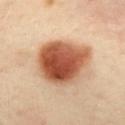Captured during whole-body skin photography for melanoma surveillance; the lesion was not biopsied.